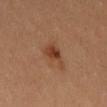The lesion-visualizer software estimated a lesion area of about 5.5 mm² and an outline eccentricity of about 0.45 (0 = round, 1 = elongated). It also reported an average lesion color of about L≈40 a*≈22 b*≈31 (CIELAB), about 9 CIELAB-L* units darker than the surrounding skin, and a normalized lesion–skin contrast near 8. And it measured a border-irregularity rating of about 3.5/10, a within-lesion color-variation index near 3.5/10, and a peripheral color-asymmetry measure near 1.5.
A roughly 15 mm field-of-view crop from a total-body skin photograph.
The patient is a female in their mid-30s.
From the left thigh.
The lesion's longest dimension is about 3 mm.